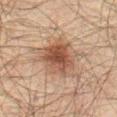Part of a total-body skin-imaging series; this lesion was reviewed on a skin check and was not flagged for biopsy. From the abdomen. The tile uses cross-polarized illumination. A close-up tile cropped from a whole-body skin photograph, about 15 mm across. A male subject, aged around 60. The lesion-visualizer software estimated a lesion area of about 17 mm² and a shape eccentricity near 0.5. And it measured a border-irregularity rating of about 2.5/10, a color-variation rating of about 4.5/10, and radial color variation of about 1. The analysis additionally found an automated nevus-likeness rating near 90 out of 100.